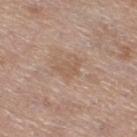{"lesion_size": {"long_diameter_mm_approx": 3.0}, "site": "leg", "patient": {"sex": "female", "age_approx": 65}, "image": {"source": "total-body photography crop", "field_of_view_mm": 15}, "lighting": "white-light"}